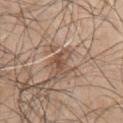Case summary:
* notes · imaged on a skin check; not biopsied
* image source · 15 mm crop, total-body photography
* automated lesion analysis · a footprint of about 5 mm² and a shape-asymmetry score of about 0.45 (0 = symmetric); a nevus-likeness score of about 95/100
* lighting · white-light
* patient · male, aged 43 to 47
* location · the right upper arm